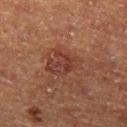This lesion was catalogued during total-body skin photography and was not selected for biopsy.
About 4 mm across.
Captured under cross-polarized illumination.
Automated tile analysis of the lesion measured a footprint of about 10 mm², an outline eccentricity of about 0.7 (0 = round, 1 = elongated), and a symmetry-axis asymmetry near 0.3. The analysis additionally found a border-irregularity rating of about 3.5/10, internal color variation of about 4 on a 0–10 scale, and radial color variation of about 1.5. The analysis additionally found a nevus-likeness score of about 5/100.
A male subject aged 73 to 77.
Located on the left thigh.
A roughly 15 mm field-of-view crop from a total-body skin photograph.